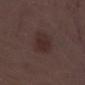Q: Is there a histopathology result?
A: imaged on a skin check; not biopsied
Q: How was this image acquired?
A: total-body-photography crop, ~15 mm field of view
Q: Patient demographics?
A: male, aged 68–72
Q: Lesion location?
A: the right lower leg
Q: How was the tile lit?
A: white-light
Q: What did automated image analysis measure?
A: an area of roughly 8.5 mm², an eccentricity of roughly 0.55, and a symmetry-axis asymmetry near 0.2; a border-irregularity rating of about 2/10 and a peripheral color-asymmetry measure near 0.5